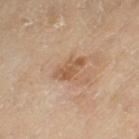Captured during whole-body skin photography for melanoma surveillance; the lesion was not biopsied. From the leg. The total-body-photography lesion software estimated a lesion area of about 6.5 mm², a shape eccentricity near 0.85, and a symmetry-axis asymmetry near 0.4. The software also gave an average lesion color of about L≈56 a*≈20 b*≈34 (CIELAB), about 9 CIELAB-L* units darker than the surrounding skin, and a normalized border contrast of about 7. The software also gave radial color variation of about 1.5. The analysis additionally found a nevus-likeness score of about 0/100 and a detector confidence of about 100 out of 100 that the crop contains a lesion. Captured under cross-polarized illumination. A male patient aged approximately 65. Measured at roughly 4 mm in maximum diameter. This image is a 15 mm lesion crop taken from a total-body photograph.